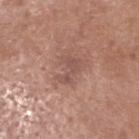| key | value |
|---|---|
| follow-up | no biopsy performed (imaged during a skin exam) |
| acquisition | total-body-photography crop, ~15 mm field of view |
| subject | male, about 50 years old |
| location | the head or neck |
| lesion size | ≈3 mm |